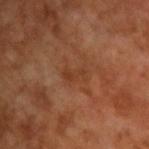image = total-body-photography crop, ~15 mm field of view | subject = male, aged 63–67 | image-analysis metrics = a lesion color around L≈37 a*≈23 b*≈32 in CIELAB and a normalized lesion–skin contrast near 5; a nevus-likeness score of about 0/100 and lesion-presence confidence of about 100/100 | size = about 2.5 mm | lighting = cross-polarized illumination.